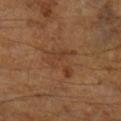This lesion was catalogued during total-body skin photography and was not selected for biopsy. A 15 mm close-up tile from a total-body photography series done for melanoma screening. A male patient aged around 65. Longest diameter approximately 5 mm.